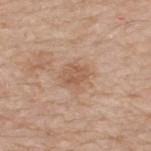notes — no biopsy performed (imaged during a skin exam)
lighting — white-light illumination
lesion diameter — ≈3 mm
anatomic site — the back
TBP lesion metrics — an area of roughly 4.5 mm², a shape eccentricity near 0.7, and two-axis asymmetry of about 0.2; about 8 CIELAB-L* units darker than the surrounding skin; border irregularity of about 2.5 on a 0–10 scale; a classifier nevus-likeness of about 0/100 and a detector confidence of about 100 out of 100 that the crop contains a lesion
subject — male, aged 63–67
image — total-body-photography crop, ~15 mm field of view